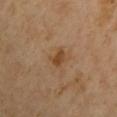The lesion was photographed on a routine skin check and not biopsied; there is no pathology result.
A lesion tile, about 15 mm wide, cut from a 3D total-body photograph.
A male subject in their mid-50s.
This is a cross-polarized tile.
The lesion is located on the left upper arm.
Automated image analysis of the tile measured an area of roughly 3.5 mm², an outline eccentricity of about 0.7 (0 = round, 1 = elongated), and a shape-asymmetry score of about 0.35 (0 = symmetric).